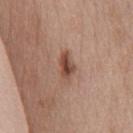The total-body-photography lesion software estimated a footprint of about 5.5 mm², a shape eccentricity near 0.85, and a symmetry-axis asymmetry near 0.25. The analysis additionally found a lesion color around L≈48 a*≈21 b*≈27 in CIELAB, roughly 12 lightness units darker than nearby skin, and a lesion-to-skin contrast of about 9 (normalized; higher = more distinct).
Located on the chest.
This is a white-light tile.
A close-up tile cropped from a whole-body skin photograph, about 15 mm across.
The patient is a female aged 38–42.